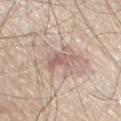Case summary:
– follow-up — catalogued during a skin exam; not biopsied
– patient — male, aged around 70
– location — the chest
– image — 15 mm crop, total-body photography
– lighting — white-light illumination
– lesion size — ≈3.5 mm